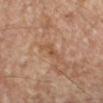subject=female, roughly 65 years of age
anatomic site=the right forearm
acquisition=~15 mm tile from a whole-body skin photo